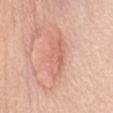follow-up: imaged on a skin check; not biopsied
site: the abdomen
illumination: white-light
automated metrics: an area of roughly 11 mm², a shape eccentricity near 0.9, and a shape-asymmetry score of about 0.25 (0 = symmetric); a lesion–skin lightness drop of about 8 and a lesion-to-skin contrast of about 5 (normalized; higher = more distinct)
subject: female, about 65 years old
image: 15 mm crop, total-body photography
lesion diameter: about 6 mm